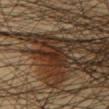Impression: This lesion was catalogued during total-body skin photography and was not selected for biopsy. Context: Longest diameter approximately 3 mm. The lesion is on the chest. The patient is a male approximately 50 years of age. Cropped from a whole-body photographic skin survey; the tile spans about 15 mm. This is a cross-polarized tile. Automated image analysis of the tile measured a footprint of about 3.5 mm². The analysis additionally found roughly 7 lightness units darker than nearby skin and a normalized border contrast of about 7.5. The analysis additionally found a border-irregularity index near 6/10, internal color variation of about 1 on a 0–10 scale, and radial color variation of about 0.5. It also reported a classifier nevus-likeness of about 0/100 and a lesion-detection confidence of about 0/100.